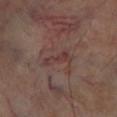Impression: The lesion was tiled from a total-body skin photograph and was not biopsied. Clinical summary: This is a cross-polarized tile. The total-body-photography lesion software estimated an eccentricity of roughly 0.95 and a shape-asymmetry score of about 0.4 (0 = symmetric). The analysis additionally found internal color variation of about 2 on a 0–10 scale. The analysis additionally found a classifier nevus-likeness of about 0/100. A roughly 15 mm field-of-view crop from a total-body skin photograph. On the leg. The recorded lesion diameter is about 4 mm.